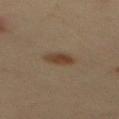Q: Is there a histopathology result?
A: total-body-photography surveillance lesion; no biopsy
Q: Who is the patient?
A: male, approximately 40 years of age
Q: What is the anatomic site?
A: the mid back
Q: What kind of image is this?
A: ~15 mm crop, total-body skin-cancer survey